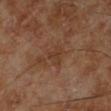Q: Was this lesion biopsied?
A: total-body-photography surveillance lesion; no biopsy
Q: What is the imaging modality?
A: ~15 mm crop, total-body skin-cancer survey
Q: Lesion location?
A: the left lower leg
Q: What is the lesion's diameter?
A: ~2.5 mm (longest diameter)
Q: What lighting was used for the tile?
A: cross-polarized illumination
Q: What are the patient's age and sex?
A: male, roughly 70 years of age
Q: Automated lesion metrics?
A: a lesion area of about 4 mm² and two-axis asymmetry of about 0.5; internal color variation of about 2 on a 0–10 scale; a classifier nevus-likeness of about 0/100 and a lesion-detection confidence of about 100/100